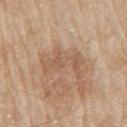Impression:
No biopsy was performed on this lesion — it was imaged during a full skin examination and was not determined to be concerning.
Context:
A male subject, aged approximately 80. This image is a 15 mm lesion crop taken from a total-body photograph. Approximately 5 mm at its widest. Located on the right upper arm.